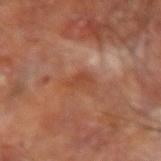No biopsy was performed on this lesion — it was imaged during a full skin examination and was not determined to be concerning. A male patient, roughly 65 years of age. A close-up tile cropped from a whole-body skin photograph, about 15 mm across. Located on the right forearm. Imaged with cross-polarized lighting.